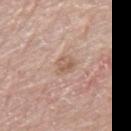workup: imaged on a skin check; not biopsied
lighting: white-light
site: the right thigh
diameter: ~2.5 mm (longest diameter)
subject: female, approximately 70 years of age
image source: 15 mm crop, total-body photography